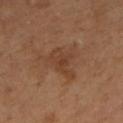biopsy_status: not biopsied; imaged during a skin examination
site: left lower leg
image:
  source: total-body photography crop
  field_of_view_mm: 15
lighting: cross-polarized
lesion_size:
  long_diameter_mm_approx: 4.5
patient:
  sex: female
  age_approx: 65
automated_metrics:
  nevus_likeness_0_100: 0
  lesion_detection_confidence_0_100: 100